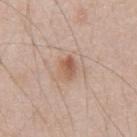Assessment:
The lesion was photographed on a routine skin check and not biopsied; there is no pathology result.
Background:
The lesion is on the abdomen. Imaged with white-light lighting. The recorded lesion diameter is about 3 mm. A male subject, aged 43–47. A 15 mm crop from a total-body photograph taken for skin-cancer surveillance.